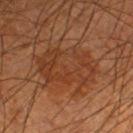Clinical impression:
No biopsy was performed on this lesion — it was imaged during a full skin examination and was not determined to be concerning.
Clinical summary:
A close-up tile cropped from a whole-body skin photograph, about 15 mm across. Approximately 7 mm at its widest. The lesion is on the left lower leg. The total-body-photography lesion software estimated a lesion area of about 23 mm² and an eccentricity of roughly 0.75. It also reported a within-lesion color-variation index near 4/10. The software also gave a classifier nevus-likeness of about 65/100 and a lesion-detection confidence of about 100/100. This is a cross-polarized tile. The subject is a male aged 53–57.